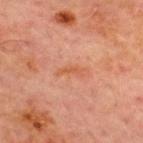Clinical impression:
This lesion was catalogued during total-body skin photography and was not selected for biopsy.
Clinical summary:
Captured under cross-polarized illumination. The lesion is located on the chest. The subject is a male aged 68 to 72. The recorded lesion diameter is about 3 mm. A lesion tile, about 15 mm wide, cut from a 3D total-body photograph.